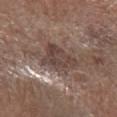Measured at roughly 4 mm in maximum diameter. The total-body-photography lesion software estimated a lesion color around L≈42 a*≈15 b*≈21 in CIELAB, about 10 CIELAB-L* units darker than the surrounding skin, and a lesion-to-skin contrast of about 8 (normalized; higher = more distinct). It also reported a border-irregularity index near 4/10, a within-lesion color-variation index near 4/10, and radial color variation of about 1.5. And it measured an automated nevus-likeness rating near 0 out of 100 and a detector confidence of about 85 out of 100 that the crop contains a lesion. A male subject aged 53–57. A 15 mm close-up extracted from a 3D total-body photography capture. This is a white-light tile. The lesion is on the arm.